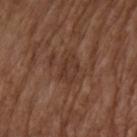Context:
On the right upper arm. A male subject, in their mid-70s. Longest diameter approximately 4 mm. Automated image analysis of the tile measured an area of roughly 7 mm² and a shape-asymmetry score of about 0.25 (0 = symmetric). And it measured an average lesion color of about L≈35 a*≈19 b*≈26 (CIELAB) and about 6 CIELAB-L* units darker than the surrounding skin. And it measured internal color variation of about 3 on a 0–10 scale and radial color variation of about 1. Imaged with white-light lighting. Cropped from a total-body skin-imaging series; the visible field is about 15 mm.